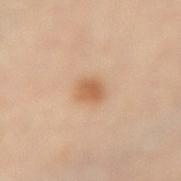- diameter · about 2.5 mm
- image · ~15 mm tile from a whole-body skin photo
- body site · the right forearm
- patient · male, aged around 55